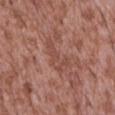  biopsy_status: not biopsied; imaged during a skin examination
  lesion_size:
    long_diameter_mm_approx: 4.5
  automated_metrics:
    shape_asymmetry: 0.55
    border_irregularity_0_10: 7.5
    peripheral_color_asymmetry: 0.5
  lighting: white-light
  patient:
    sex: male
    age_approx: 45
  image:
    source: total-body photography crop
    field_of_view_mm: 15
  site: abdomen